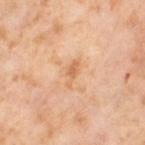The lesion was photographed on a routine skin check and not biopsied; there is no pathology result. Located on the leg. Automated tile analysis of the lesion measured an outline eccentricity of about 0.9 (0 = round, 1 = elongated). The analysis additionally found a border-irregularity rating of about 4/10, a color-variation rating of about 1/10, and radial color variation of about 0. The patient is a female aged 53 to 57. Cropped from a total-body skin-imaging series; the visible field is about 15 mm. This is a cross-polarized tile.